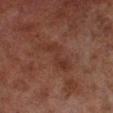This lesion was catalogued during total-body skin photography and was not selected for biopsy. A roughly 15 mm field-of-view crop from a total-body skin photograph. About 5.5 mm across. The lesion is located on the right lower leg. A male subject approximately 70 years of age.